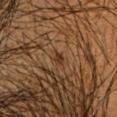Recorded during total-body skin imaging; not selected for excision or biopsy.
An algorithmic analysis of the crop reported an area of roughly 1.5 mm² and two-axis asymmetry of about 0.25. The software also gave a classifier nevus-likeness of about 60/100 and lesion-presence confidence of about 90/100.
A 15 mm close-up tile from a total-body photography series done for melanoma screening.
Approximately 1.5 mm at its widest.
On the head or neck.
The tile uses cross-polarized illumination.
A male subject about 50 years old.